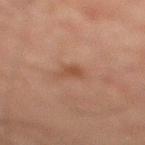Captured under cross-polarized illumination.
On the right lower leg.
A 15 mm close-up extracted from a 3D total-body photography capture.
The patient is a male about 30 years old.